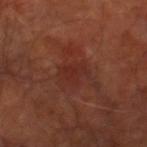Impression:
Imaged during a routine full-body skin examination; the lesion was not biopsied and no histopathology is available.
Context:
Cropped from a whole-body photographic skin survey; the tile spans about 15 mm. The subject is aged approximately 65. The recorded lesion diameter is about 3 mm. The tile uses cross-polarized illumination. From the leg.